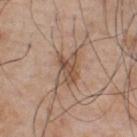workup: imaged on a skin check; not biopsied
lesion size: ~4.5 mm (longest diameter)
illumination: white-light
acquisition: ~15 mm tile from a whole-body skin photo
automated lesion analysis: a classifier nevus-likeness of about 5/100
body site: the chest
subject: male, roughly 50 years of age